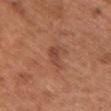biopsy status — no biopsy performed (imaged during a skin exam)
subject — female, about 65 years old
tile lighting — white-light illumination
image-analysis metrics — an eccentricity of roughly 0.8 and two-axis asymmetry of about 0.45; an average lesion color of about L≈46 a*≈24 b*≈30 (CIELAB) and about 8 CIELAB-L* units darker than the surrounding skin
size — about 3 mm
site — the front of the torso
image source — ~15 mm crop, total-body skin-cancer survey